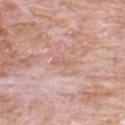Clinical impression: This lesion was catalogued during total-body skin photography and was not selected for biopsy. Image and clinical context: Cropped from a whole-body photographic skin survey; the tile spans about 15 mm. The recorded lesion diameter is about 3 mm. From the chest. The tile uses white-light illumination. The patient is a male aged approximately 80. An algorithmic analysis of the crop reported a lesion color around L≈63 a*≈20 b*≈29 in CIELAB and about 6 CIELAB-L* units darker than the surrounding skin. The analysis additionally found border irregularity of about 3.5 on a 0–10 scale and a color-variation rating of about 0.5/10.